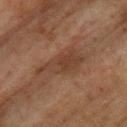biopsy status — total-body-photography surveillance lesion; no biopsy | image-analysis metrics — an area of roughly 11 mm², a shape eccentricity near 0.9, and two-axis asymmetry of about 0.4; an average lesion color of about L≈32 a*≈17 b*≈24 (CIELAB), about 6 CIELAB-L* units darker than the surrounding skin, and a normalized lesion–skin contrast near 6.5; border irregularity of about 5 on a 0–10 scale and a color-variation rating of about 3/10; an automated nevus-likeness rating near 0 out of 100 and a lesion-detection confidence of about 95/100 | image — total-body-photography crop, ~15 mm field of view | subject — male, aged around 75 | site — the upper back | tile lighting — cross-polarized illumination | lesion diameter — about 6 mm.